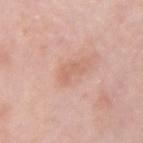{"biopsy_status": "not biopsied; imaged during a skin examination", "site": "left forearm", "patient": {"sex": "female", "age_approx": 65}, "image": {"source": "total-body photography crop", "field_of_view_mm": 15}, "lesion_size": {"long_diameter_mm_approx": 2.5}, "lighting": "white-light"}